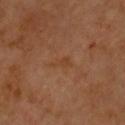Part of a total-body skin-imaging series; this lesion was reviewed on a skin check and was not flagged for biopsy. On the chest. Approximately 2.5 mm at its widest. The tile uses cross-polarized illumination. This image is a 15 mm lesion crop taken from a total-body photograph. A male subject, aged 63–67.